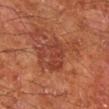Part of a total-body skin-imaging series; this lesion was reviewed on a skin check and was not flagged for biopsy. A region of skin cropped from a whole-body photographic capture, roughly 15 mm wide. A male subject, approximately 65 years of age. Located on the right lower leg.